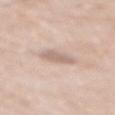Q: Is there a histopathology result?
A: imaged on a skin check; not biopsied
Q: How was the tile lit?
A: white-light
Q: What did automated image analysis measure?
A: a lesion area of about 5.5 mm² and an eccentricity of roughly 0.8; a lesion color around L≈65 a*≈16 b*≈24 in CIELAB and about 9 CIELAB-L* units darker than the surrounding skin; a border-irregularity rating of about 2/10, a within-lesion color-variation index near 1/10, and peripheral color asymmetry of about 0.5; a nevus-likeness score of about 0/100 and a detector confidence of about 95 out of 100 that the crop contains a lesion
Q: What are the patient's age and sex?
A: male, aged 78–82
Q: What is the lesion's diameter?
A: about 3.5 mm
Q: What is the imaging modality?
A: ~15 mm crop, total-body skin-cancer survey
Q: Lesion location?
A: the mid back